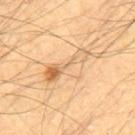No biopsy was performed on this lesion — it was imaged during a full skin examination and was not determined to be concerning. On the mid back. The lesion's longest dimension is about 6 mm. This is a cross-polarized tile. A male patient, in their mid- to late 50s. Cropped from a whole-body photographic skin survey; the tile spans about 15 mm.